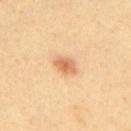follow-up = no biopsy performed (imaged during a skin exam)
location = the upper back
patient = male, aged approximately 40
lighting = cross-polarized
image source = 15 mm crop, total-body photography
lesion size = about 3 mm